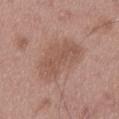Case summary:
- workup · no biopsy performed (imaged during a skin exam)
- image source · ~15 mm tile from a whole-body skin photo
- lighting · white-light
- location · the left thigh
- patient · male, aged 48 to 52
- size · ≈6 mm